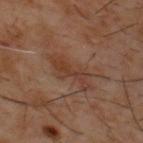Q: How was this image acquired?
A: total-body-photography crop, ~15 mm field of view
Q: Who is the patient?
A: male, about 60 years old
Q: How large is the lesion?
A: ~6.5 mm (longest diameter)
Q: Where on the body is the lesion?
A: the upper back
Q: Illumination type?
A: cross-polarized